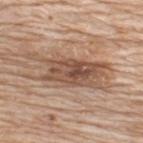follow-up: imaged on a skin check; not biopsied.